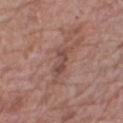Impression:
Captured during whole-body skin photography for melanoma surveillance; the lesion was not biopsied.
Clinical summary:
The lesion is on the right thigh. This image is a 15 mm lesion crop taken from a total-body photograph. Automated image analysis of the tile measured a mean CIELAB color near L≈47 a*≈20 b*≈24, about 8 CIELAB-L* units darker than the surrounding skin, and a normalized lesion–skin contrast near 6.5. This is a white-light tile. A female patient approximately 70 years of age.